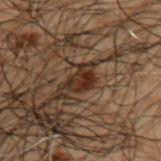Impression: Part of a total-body skin-imaging series; this lesion was reviewed on a skin check and was not flagged for biopsy. Background: Located on the upper back. Imaged with cross-polarized lighting. A male subject, approximately 50 years of age. The lesion's longest dimension is about 3 mm. A region of skin cropped from a whole-body photographic capture, roughly 15 mm wide.